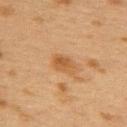Clinical impression: Recorded during total-body skin imaging; not selected for excision or biopsy. Context: A region of skin cropped from a whole-body photographic capture, roughly 15 mm wide. A female subject roughly 40 years of age. The lesion is on the back.